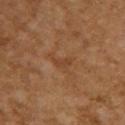The lesion was tiled from a total-body skin photograph and was not biopsied. Captured under cross-polarized illumination. Automated tile analysis of the lesion measured a footprint of about 4 mm², a shape eccentricity near 0.8, and a shape-asymmetry score of about 0.55 (0 = symmetric). The software also gave a classifier nevus-likeness of about 0/100 and a lesion-detection confidence of about 100/100. A female patient, aged 58–62. Cropped from a whole-body photographic skin survey; the tile spans about 15 mm. The lesion is on the upper back.